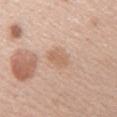{
  "biopsy_status": "not biopsied; imaged during a skin examination",
  "patient": {
    "sex": "female",
    "age_approx": 40
  },
  "site": "right upper arm",
  "image": {
    "source": "total-body photography crop",
    "field_of_view_mm": 15
  },
  "automated_metrics": {
    "area_mm2_approx": 5.5,
    "eccentricity": 0.8,
    "shape_asymmetry": 0.15,
    "border_irregularity_0_10": 1.5,
    "color_variation_0_10": 2.0,
    "peripheral_color_asymmetry": 0.5
  },
  "lighting": "white-light"
}